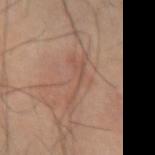This lesion was catalogued during total-body skin photography and was not selected for biopsy. A close-up tile cropped from a whole-body skin photograph, about 15 mm across. The lesion's longest dimension is about 5.5 mm. A male patient, approximately 65 years of age. On the left forearm. Imaged with cross-polarized lighting.